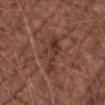workup: imaged on a skin check; not biopsied
anatomic site: the right forearm
lighting: white-light illumination
subject: male, aged 68 to 72
image source: ~15 mm crop, total-body skin-cancer survey
lesion size: about 5.5 mm
image-analysis metrics: a footprint of about 8 mm², an outline eccentricity of about 0.95 (0 = round, 1 = elongated), and a symmetry-axis asymmetry near 0.5; a classifier nevus-likeness of about 0/100 and a lesion-detection confidence of about 55/100